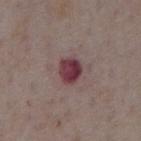The lesion was tiled from a total-body skin photograph and was not biopsied.
On the abdomen.
A lesion tile, about 15 mm wide, cut from a 3D total-body photograph.
A male subject, about 75 years old.